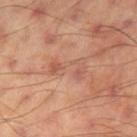<record>
  <biopsy_status>not biopsied; imaged during a skin examination</biopsy_status>
  <lesion_size>
    <long_diameter_mm_approx>4.0</long_diameter_mm_approx>
  </lesion_size>
  <patient>
    <sex>male</sex>
    <age_approx>45</age_approx>
  </patient>
  <image>
    <source>total-body photography crop</source>
    <field_of_view_mm>15</field_of_view_mm>
  </image>
  <site>right thigh</site>
  <lighting>cross-polarized</lighting>
</record>